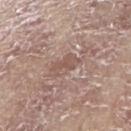Case summary:
- tile lighting: white-light illumination
- patient: male, approximately 65 years of age
- anatomic site: the right forearm
- TBP lesion metrics: an eccentricity of roughly 0.8 and a shape-asymmetry score of about 0.3 (0 = symmetric); an average lesion color of about L≈53 a*≈18 b*≈23 (CIELAB), about 7 CIELAB-L* units darker than the surrounding skin, and a normalized lesion–skin contrast near 5; border irregularity of about 3.5 on a 0–10 scale, a within-lesion color-variation index near 2/10, and a peripheral color-asymmetry measure near 0.5
- lesion size: ~3.5 mm (longest diameter)
- acquisition: 15 mm crop, total-body photography